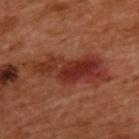Impression:
The lesion was tiled from a total-body skin photograph and was not biopsied.
Image and clinical context:
A male subject, in their 50s. Longest diameter approximately 9 mm. From the back. Captured under cross-polarized illumination. A 15 mm close-up tile from a total-body photography series done for melanoma screening. The total-body-photography lesion software estimated an outline eccentricity of about 0.95 (0 = round, 1 = elongated). The software also gave a lesion color around L≈33 a*≈28 b*≈29 in CIELAB, roughly 10 lightness units darker than nearby skin, and a lesion-to-skin contrast of about 8.5 (normalized; higher = more distinct). The software also gave a classifier nevus-likeness of about 0/100 and a lesion-detection confidence of about 95/100.